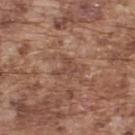| feature | finding |
|---|---|
| biopsy status | no biopsy performed (imaged during a skin exam) |
| lesion diameter | ~2.5 mm (longest diameter) |
| image source | ~15 mm crop, total-body skin-cancer survey |
| patient | male, approximately 75 years of age |
| illumination | white-light |
| location | the upper back |